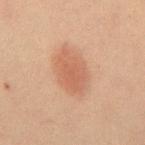<tbp_lesion>
  <biopsy_status>not biopsied; imaged during a skin examination</biopsy_status>
  <image>
    <source>total-body photography crop</source>
    <field_of_view_mm>15</field_of_view_mm>
  </image>
  <patient>
    <sex>male</sex>
    <age_approx>60</age_approx>
  </patient>
  <site>back</site>
  <lesion_size>
    <long_diameter_mm_approx>5.5</long_diameter_mm_approx>
  </lesion_size>
  <lighting>cross-polarized</lighting>
</tbp_lesion>